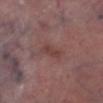The lesion was photographed on a routine skin check and not biopsied; there is no pathology result.
On the right lower leg.
A male patient, aged approximately 40.
A lesion tile, about 15 mm wide, cut from a 3D total-body photograph.
Approximately 3 mm at its widest.